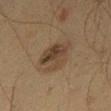<lesion>
  <biopsy_status>not biopsied; imaged during a skin examination</biopsy_status>
  <automated_metrics>
    <area_mm2_approx>11.0</area_mm2_approx>
    <eccentricity>0.25</eccentricity>
    <shape_asymmetry>0.3</shape_asymmetry>
    <border_irregularity_0_10>3.0</border_irregularity_0_10>
    <color_variation_0_10>5.0</color_variation_0_10>
    <peripheral_color_asymmetry>2.0</peripheral_color_asymmetry>
    <nevus_likeness_0_100>40</nevus_likeness_0_100>
    <lesion_detection_confidence_0_100>100</lesion_detection_confidence_0_100>
  </automated_metrics>
  <lighting>cross-polarized</lighting>
  <site>left thigh</site>
  <patient>
    <sex>male</sex>
    <age_approx>60</age_approx>
  </patient>
  <image>
    <source>total-body photography crop</source>
    <field_of_view_mm>15</field_of_view_mm>
  </image>
  <lesion_size>
    <long_diameter_mm_approx>4.0</long_diameter_mm_approx>
  </lesion_size>
</lesion>